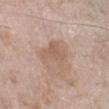{"biopsy_status": "not biopsied; imaged during a skin examination", "image": {"source": "total-body photography crop", "field_of_view_mm": 15}, "site": "left lower leg", "patient": {"sex": "female", "age_approx": 75}}